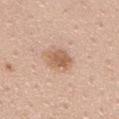<tbp_lesion>
  <biopsy_status>not biopsied; imaged during a skin examination</biopsy_status>
  <site>mid back</site>
  <lighting>white-light</lighting>
  <patient>
    <sex>male</sex>
    <age_approx>60</age_approx>
  </patient>
  <lesion_size>
    <long_diameter_mm_approx>3.0</long_diameter_mm_approx>
  </lesion_size>
  <automated_metrics>
    <border_irregularity_0_10>2.5</border_irregularity_0_10>
    <color_variation_0_10>3.5</color_variation_0_10>
    <peripheral_color_asymmetry>1.0</peripheral_color_asymmetry>
    <nevus_likeness_0_100>55</nevus_likeness_0_100>
    <lesion_detection_confidence_0_100>100</lesion_detection_confidence_0_100>
  </automated_metrics>
  <image>
    <source>total-body photography crop</source>
    <field_of_view_mm>15</field_of_view_mm>
  </image>
</tbp_lesion>